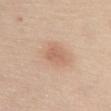diameter: ≈3 mm; anatomic site: the abdomen; image: 15 mm crop, total-body photography; illumination: white-light illumination; patient: female, roughly 70 years of age.